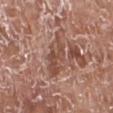Automated image analysis of the tile measured an area of roughly 10 mm², a shape eccentricity near 0.85, and a symmetry-axis asymmetry near 0.2. The software also gave internal color variation of about 4 on a 0–10 scale. A male patient, aged 73–77. The lesion's longest dimension is about 5.5 mm. From the right lower leg. This is a white-light tile. A close-up tile cropped from a whole-body skin photograph, about 15 mm across.